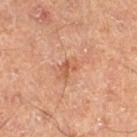biopsy status — no biopsy performed (imaged during a skin exam); anatomic site — the right thigh; tile lighting — cross-polarized illumination; subject — male, in their mid- to late 60s; automated lesion analysis — a lesion area of about 4 mm², an outline eccentricity of about 0.8 (0 = round, 1 = elongated), and a symmetry-axis asymmetry near 0.3; lesion size — about 3 mm; image source — total-body-photography crop, ~15 mm field of view.